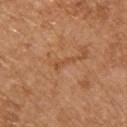Impression:
Recorded during total-body skin imaging; not selected for excision or biopsy.
Acquisition and patient details:
An algorithmic analysis of the crop reported an area of roughly 2 mm² and an outline eccentricity of about 0.95 (0 = round, 1 = elongated). And it measured a lesion color around L≈51 a*≈24 b*≈37 in CIELAB, about 7 CIELAB-L* units darker than the surrounding skin, and a normalized lesion–skin contrast near 5. It also reported a border-irregularity index near 6/10, a within-lesion color-variation index near 0/10, and radial color variation of about 0. A male subject, about 70 years old. A roughly 15 mm field-of-view crop from a total-body skin photograph. Longest diameter approximately 3 mm. On the chest. The tile uses white-light illumination.